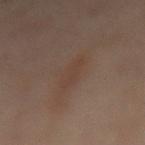body site = the mid back | lesion size = about 5 mm | imaging modality = ~15 mm crop, total-body skin-cancer survey | automated metrics = an average lesion color of about L≈32 a*≈13 b*≈21 (CIELAB), about 4 CIELAB-L* units darker than the surrounding skin, and a normalized lesion–skin contrast near 4.5; a classifier nevus-likeness of about 5/100 and a lesion-detection confidence of about 95/100 | lighting = cross-polarized illumination | patient = female, aged 53 to 57.